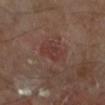  biopsy_status: not biopsied; imaged during a skin examination
  image:
    source: total-body photography crop
    field_of_view_mm: 15
  lighting: cross-polarized
  automated_metrics:
    shape_asymmetry: 0.3
    cielab_L: 34
    cielab_a: 20
    cielab_b: 22
    vs_skin_darker_L: 5.0
    border_irregularity_0_10: 2.5
    color_variation_0_10: 2.0
    peripheral_color_asymmetry: 0.5
  site: leg
  lesion_size:
    long_diameter_mm_approx: 2.5
  patient:
    sex: male
    age_approx: 70